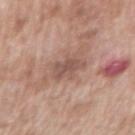Clinical impression: Recorded during total-body skin imaging; not selected for excision or biopsy. Acquisition and patient details: The patient is a male about 70 years old. The lesion's longest dimension is about 3.5 mm. The lesion-visualizer software estimated an area of roughly 6 mm² and two-axis asymmetry of about 0.3. It also reported border irregularity of about 3.5 on a 0–10 scale, internal color variation of about 2 on a 0–10 scale, and a peripheral color-asymmetry measure near 0.5. The software also gave a classifier nevus-likeness of about 0/100. The lesion is on the right upper arm. Cropped from a whole-body photographic skin survey; the tile spans about 15 mm.